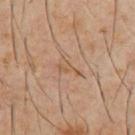Acquisition and patient details:
Captured under cross-polarized illumination. Measured at roughly 3 mm in maximum diameter. An algorithmic analysis of the crop reported an area of roughly 3.5 mm². It also reported an average lesion color of about L≈51 a*≈17 b*≈30 (CIELAB). The analysis additionally found a classifier nevus-likeness of about 0/100 and a lesion-detection confidence of about 90/100. The lesion is located on the front of the torso. A male patient, aged 58–62. A close-up tile cropped from a whole-body skin photograph, about 15 mm across.